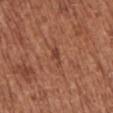No biopsy was performed on this lesion — it was imaged during a full skin examination and was not determined to be concerning. A 15 mm crop from a total-body photograph taken for skin-cancer surveillance. A female patient aged 73 to 77. Captured under white-light illumination. Located on the left upper arm. The recorded lesion diameter is about 2.5 mm. The total-body-photography lesion software estimated a lesion color around L≈43 a*≈25 b*≈30 in CIELAB, a lesion–skin lightness drop of about 8, and a lesion-to-skin contrast of about 6 (normalized; higher = more distinct). And it measured a border-irregularity index near 3.5/10, a within-lesion color-variation index near 1/10, and peripheral color asymmetry of about 0.5. It also reported a detector confidence of about 95 out of 100 that the crop contains a lesion.